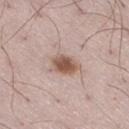Clinical impression:
Recorded during total-body skin imaging; not selected for excision or biopsy.
Background:
A close-up tile cropped from a whole-body skin photograph, about 15 mm across. A male subject aged around 50. Longest diameter approximately 3.5 mm. Located on the right thigh.